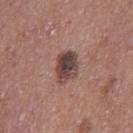Assessment: This lesion was catalogued during total-body skin photography and was not selected for biopsy. Clinical summary: Measured at roughly 3.5 mm in maximum diameter. A female patient approximately 40 years of age. Automated image analysis of the tile measured a mean CIELAB color near L≈42 a*≈18 b*≈20 and a normalized lesion–skin contrast near 11. Captured under white-light illumination. A 15 mm close-up extracted from a 3D total-body photography capture. On the left thigh.